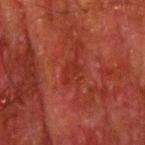illumination: cross-polarized illumination | TBP lesion metrics: an area of roughly 4.5 mm², a shape eccentricity near 0.7, and a shape-asymmetry score of about 0.35 (0 = symmetric); a lesion color around L≈26 a*≈29 b*≈27 in CIELAB, about 5 CIELAB-L* units darker than the surrounding skin, and a normalized lesion–skin contrast near 5; a border-irregularity index near 4/10 and radial color variation of about 0.5 | lesion size: ~3 mm (longest diameter) | image source: 15 mm crop, total-body photography | subject: male, aged 78 to 82 | body site: the right upper arm.